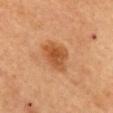Part of a total-body skin-imaging series; this lesion was reviewed on a skin check and was not flagged for biopsy. This is a cross-polarized tile. A roughly 15 mm field-of-view crop from a total-body skin photograph. Located on the back. The lesion-visualizer software estimated a detector confidence of about 100 out of 100 that the crop contains a lesion. A female subject, aged around 60.